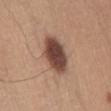Captured during whole-body skin photography for melanoma surveillance; the lesion was not biopsied.
The lesion is on the abdomen.
Approximately 5.5 mm at its widest.
The lesion-visualizer software estimated a mean CIELAB color near L≈45 a*≈20 b*≈25, roughly 17 lightness units darker than nearby skin, and a normalized border contrast of about 12.5. The analysis additionally found a border-irregularity index near 1.5/10, a within-lesion color-variation index near 5.5/10, and peripheral color asymmetry of about 1.5.
Imaged with white-light lighting.
A lesion tile, about 15 mm wide, cut from a 3D total-body photograph.
The subject is a male in their mid- to late 30s.